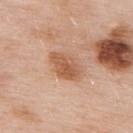workup: imaged on a skin check; not biopsied
TBP lesion metrics: a footprint of about 9 mm², an outline eccentricity of about 0.85 (0 = round, 1 = elongated), and a symmetry-axis asymmetry near 0.15; border irregularity of about 2 on a 0–10 scale and radial color variation of about 1
site: the upper back
imaging modality: total-body-photography crop, ~15 mm field of view
patient: male, in their mid-50s
lighting: white-light illumination
lesion size: ~4.5 mm (longest diameter)